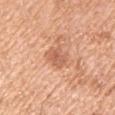Assessment:
The lesion was tiled from a total-body skin photograph and was not biopsied.
Context:
A 15 mm close-up extracted from a 3D total-body photography capture. Imaged with white-light lighting. The lesion is located on the left upper arm. A male subject aged approximately 55. Longest diameter approximately 2.5 mm.